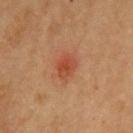The lesion was photographed on a routine skin check and not biopsied; there is no pathology result.
The lesion is located on the left upper arm.
A close-up tile cropped from a whole-body skin photograph, about 15 mm across.
This is a cross-polarized tile.
A female subject, aged 68 to 72.
Approximately 3 mm at its widest.